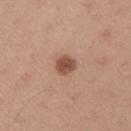Case summary:
• biopsy status — imaged on a skin check; not biopsied
• lesion diameter — ≈2.5 mm
• anatomic site — the left upper arm
• TBP lesion metrics — a footprint of about 5 mm², a shape eccentricity near 0.45, and two-axis asymmetry of about 0.15; a lesion–skin lightness drop of about 13 and a normalized border contrast of about 9; a classifier nevus-likeness of about 100/100 and a lesion-detection confidence of about 100/100
• illumination — white-light illumination
• patient — male, roughly 35 years of age
• imaging modality — 15 mm crop, total-body photography